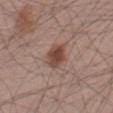Approximately 3.5 mm at its widest.
Imaged with white-light lighting.
A close-up tile cropped from a whole-body skin photograph, about 15 mm across.
The patient is a male in their mid- to late 40s.
Located on the right thigh.
Automated tile analysis of the lesion measured a footprint of about 7 mm², an eccentricity of roughly 0.65, and a symmetry-axis asymmetry near 0.2. The analysis additionally found a lesion color around L≈46 a*≈19 b*≈25 in CIELAB, roughly 11 lightness units darker than nearby skin, and a normalized border contrast of about 9.